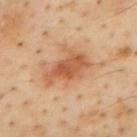biopsy_status: not biopsied; imaged during a skin examination
image:
  source: total-body photography crop
  field_of_view_mm: 15
patient:
  sex: male
  age_approx: 55
site: mid back
automated_metrics:
  area_mm2_approx: 13.0
  eccentricity: 0.8
  border_irregularity_0_10: 4.5
  color_variation_0_10: 4.0
lighting: cross-polarized